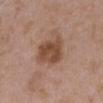Impression: Captured during whole-body skin photography for melanoma surveillance; the lesion was not biopsied. Context: A female patient in their mid-30s. Cropped from a whole-body photographic skin survey; the tile spans about 15 mm. Imaged with white-light lighting. The lesion's longest dimension is about 4.5 mm. The lesion is located on the left upper arm. The lesion-visualizer software estimated an area of roughly 12 mm², an outline eccentricity of about 0.55 (0 = round, 1 = elongated), and a symmetry-axis asymmetry near 0.2. The software also gave a mean CIELAB color near L≈47 a*≈20 b*≈29, about 11 CIELAB-L* units darker than the surrounding skin, and a normalized border contrast of about 9.